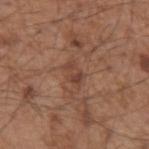Captured during whole-body skin photography for melanoma surveillance; the lesion was not biopsied. The lesion is on the left upper arm. This image is a 15 mm lesion crop taken from a total-body photograph. A male subject aged 53–57. About 3 mm across. This is a white-light tile.